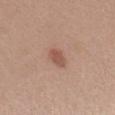notes — total-body-photography surveillance lesion; no biopsy
tile lighting — white-light
site — the chest
patient — female, approximately 35 years of age
imaging modality — total-body-photography crop, ~15 mm field of view